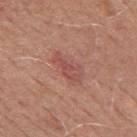<case>
  <biopsy_status>not biopsied; imaged during a skin examination</biopsy_status>
  <image>
    <source>total-body photography crop</source>
    <field_of_view_mm>15</field_of_view_mm>
  </image>
  <lesion_size>
    <long_diameter_mm_approx>4.5</long_diameter_mm_approx>
  </lesion_size>
  <lighting>white-light</lighting>
  <patient>
    <sex>male</sex>
    <age_approx>50</age_approx>
  </patient>
  <site>mid back</site>
  <automated_metrics>
    <area_mm2_approx>7.0</area_mm2_approx>
    <eccentricity>0.9</eccentricity>
    <shape_asymmetry>0.35</shape_asymmetry>
    <cielab_L>51</cielab_L>
    <cielab_a>25</cielab_a>
    <cielab_b>26</cielab_b>
    <vs_skin_darker_L>7.0</vs_skin_darker_L>
    <vs_skin_contrast_norm>5.5</vs_skin_contrast_norm>
    <border_irregularity_0_10>4.0</border_irregularity_0_10>
    <color_variation_0_10>2.5</color_variation_0_10>
    <peripheral_color_asymmetry>1.0</peripheral_color_asymmetry>
    <nevus_likeness_0_100>5</nevus_likeness_0_100>
    <lesion_detection_confidence_0_100>100</lesion_detection_confidence_0_100>
  </automated_metrics>
</case>